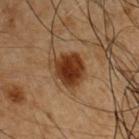| feature | finding |
|---|---|
| follow-up | catalogued during a skin exam; not biopsied |
| lighting | cross-polarized |
| image source | total-body-photography crop, ~15 mm field of view |
| lesion diameter | about 3.5 mm |
| patient | male, roughly 50 years of age |
| anatomic site | the right upper arm |